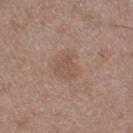Assessment: Recorded during total-body skin imaging; not selected for excision or biopsy. Background: A male patient, approximately 50 years of age. On the left lower leg. A 15 mm close-up tile from a total-body photography series done for melanoma screening.